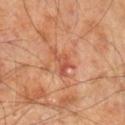Part of a total-body skin-imaging series; this lesion was reviewed on a skin check and was not flagged for biopsy.